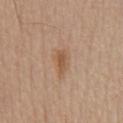{
  "biopsy_status": "not biopsied; imaged during a skin examination",
  "lesion_size": {
    "long_diameter_mm_approx": 3.0
  },
  "image": {
    "source": "total-body photography crop",
    "field_of_view_mm": 15
  },
  "automated_metrics": {
    "cielab_L": 54,
    "cielab_a": 19,
    "cielab_b": 33,
    "vs_skin_darker_L": 9.0
  },
  "lighting": "white-light",
  "patient": {
    "sex": "male",
    "age_approx": 65
  },
  "site": "chest"
}